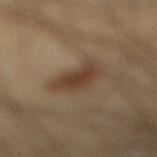| key | value |
|---|---|
| follow-up | imaged on a skin check; not biopsied |
| image source | total-body-photography crop, ~15 mm field of view |
| anatomic site | the right lower leg |
| subject | male, in their mid- to late 30s |